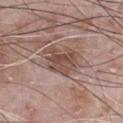Recorded during total-body skin imaging; not selected for excision or biopsy. Located on the chest. This is a white-light tile. The subject is a male aged around 70. The lesion's longest dimension is about 3.5 mm. Automated tile analysis of the lesion measured a footprint of about 8.5 mm², an outline eccentricity of about 0.55 (0 = round, 1 = elongated), and two-axis asymmetry of about 0.35. The software also gave an automated nevus-likeness rating near 15 out of 100 and a detector confidence of about 100 out of 100 that the crop contains a lesion. A 15 mm close-up extracted from a 3D total-body photography capture.